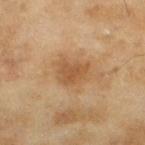– workup · catalogued during a skin exam; not biopsied
– anatomic site · the left lower leg
– diameter · ~3.5 mm (longest diameter)
– subject · female, aged 58–62
– TBP lesion metrics · a footprint of about 8.5 mm², an outline eccentricity of about 0.65 (0 = round, 1 = elongated), and a symmetry-axis asymmetry near 0.3
– tile lighting · cross-polarized illumination
– image · ~15 mm crop, total-body skin-cancer survey